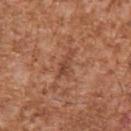{
  "biopsy_status": "not biopsied; imaged during a skin examination",
  "lighting": "white-light",
  "site": "arm",
  "patient": {
    "sex": "male",
    "age_approx": 45
  },
  "image": {
    "source": "total-body photography crop",
    "field_of_view_mm": 15
  },
  "lesion_size": {
    "long_diameter_mm_approx": 3.5
  }
}